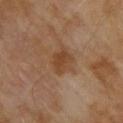  biopsy_status: not biopsied; imaged during a skin examination
  lesion_size:
    long_diameter_mm_approx: 3.0
  automated_metrics:
    area_mm2_approx: 6.5
    eccentricity: 0.65
    shape_asymmetry: 0.25
  site: upper back
  image:
    source: total-body photography crop
    field_of_view_mm: 15
  patient:
    sex: male
    age_approx: 70